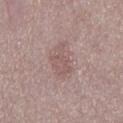Assessment: Part of a total-body skin-imaging series; this lesion was reviewed on a skin check and was not flagged for biopsy. Image and clinical context: Located on the mid back. A 15 mm close-up extracted from a 3D total-body photography capture. A male patient about 70 years old.